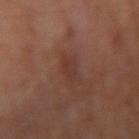Recorded during total-body skin imaging; not selected for excision or biopsy. Cropped from a whole-body photographic skin survey; the tile spans about 15 mm. The subject is about 55 years old. Automated tile analysis of the lesion measured an area of roughly 3 mm² and a shape eccentricity near 0.8. The software also gave a classifier nevus-likeness of about 5/100 and a detector confidence of about 100 out of 100 that the crop contains a lesion. Located on the right upper arm. Captured under cross-polarized illumination. Longest diameter approximately 2.5 mm.